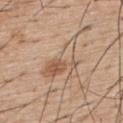Q: Was a biopsy performed?
A: imaged on a skin check; not biopsied
Q: What is the imaging modality?
A: 15 mm crop, total-body photography
Q: Who is the patient?
A: male, approximately 55 years of age
Q: How large is the lesion?
A: about 6 mm
Q: How was the tile lit?
A: white-light illumination
Q: Lesion location?
A: the back